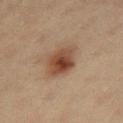workup: catalogued during a skin exam; not biopsied
lesion size: about 4 mm
image source: 15 mm crop, total-body photography
location: the right thigh
automated metrics: a lesion area of about 11 mm²; an average lesion color of about L≈39 a*≈17 b*≈26 (CIELAB), a lesion–skin lightness drop of about 11, and a lesion-to-skin contrast of about 9 (normalized; higher = more distinct); a color-variation rating of about 6.5/10 and radial color variation of about 1.5; a nevus-likeness score of about 100/100 and a detector confidence of about 100 out of 100 that the crop contains a lesion
subject: female, aged around 40
illumination: cross-polarized illumination